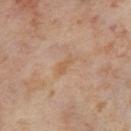A female patient in their mid- to late 50s.
Measured at roughly 3 mm in maximum diameter.
The lesion is located on the right thigh.
The tile uses cross-polarized illumination.
Cropped from a whole-body photographic skin survey; the tile spans about 15 mm.